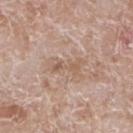Acquisition and patient details:
This is a white-light tile. A region of skin cropped from a whole-body photographic capture, roughly 15 mm wide. Longest diameter approximately 4.5 mm. The subject is a male roughly 60 years of age. The lesion is located on the left lower leg. Automated tile analysis of the lesion measured a footprint of about 6.5 mm², an eccentricity of roughly 0.9, and a symmetry-axis asymmetry near 0.4. The software also gave a classifier nevus-likeness of about 0/100 and a lesion-detection confidence of about 95/100.